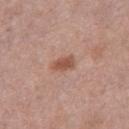Captured under white-light illumination.
About 3 mm across.
The lesion is located on the right thigh.
A female patient, roughly 40 years of age.
The total-body-photography lesion software estimated a footprint of about 4 mm² and a shape eccentricity near 0.8. The software also gave a border-irregularity rating of about 2/10, a within-lesion color-variation index near 1.5/10, and a peripheral color-asymmetry measure near 0.5. The analysis additionally found a classifier nevus-likeness of about 85/100.
This image is a 15 mm lesion crop taken from a total-body photograph.